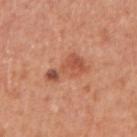Case summary:
* follow-up: catalogued during a skin exam; not biopsied
* image source: 15 mm crop, total-body photography
* lighting: white-light illumination
* anatomic site: the right upper arm
* TBP lesion metrics: roughly 10 lightness units darker than nearby skin and a lesion-to-skin contrast of about 6.5 (normalized; higher = more distinct); a border-irregularity rating of about 6/10 and peripheral color asymmetry of about 1.5; an automated nevus-likeness rating near 70 out of 100 and lesion-presence confidence of about 100/100
* size: ~5 mm (longest diameter)
* patient: male, in their mid-50s